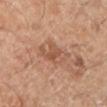Findings:
- diameter — ~4.5 mm (longest diameter)
- subject — in their mid- to late 60s
- image — total-body-photography crop, ~15 mm field of view
- anatomic site — the left lower leg